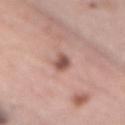Captured under white-light illumination. The subject is a male approximately 65 years of age. An algorithmic analysis of the crop reported an area of roughly 3.5 mm², a shape eccentricity near 0.85, and a symmetry-axis asymmetry near 0.35. And it measured a lesion color around L≈53 a*≈22 b*≈25 in CIELAB, roughly 14 lightness units darker than nearby skin, and a lesion-to-skin contrast of about 9.5 (normalized; higher = more distinct). The software also gave border irregularity of about 3 on a 0–10 scale, a color-variation rating of about 2.5/10, and a peripheral color-asymmetry measure near 1. The lesion's longest dimension is about 3 mm. From the mid back. A roughly 15 mm field-of-view crop from a total-body skin photograph.